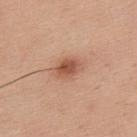| field | value |
|---|---|
| notes | total-body-photography surveillance lesion; no biopsy |
| imaging modality | ~15 mm crop, total-body skin-cancer survey |
| anatomic site | the upper back |
| patient | male, in their mid-30s |